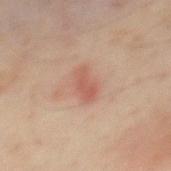Findings:
- follow-up · total-body-photography surveillance lesion; no biopsy
- subject · male, roughly 60 years of age
- image source · ~15 mm crop, total-body skin-cancer survey
- anatomic site · the mid back